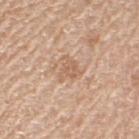Q: Was this lesion biopsied?
A: catalogued during a skin exam; not biopsied